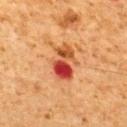Recorded during total-body skin imaging; not selected for excision or biopsy. Cropped from a whole-body photographic skin survey; the tile spans about 15 mm. The lesion is on the back. An algorithmic analysis of the crop reported a lesion area of about 11 mm², an eccentricity of roughly 0.75, and a shape-asymmetry score of about 0.25 (0 = symmetric). And it measured a within-lesion color-variation index near 10/10 and a peripheral color-asymmetry measure near 7.5. A male patient approximately 60 years of age. The tile uses cross-polarized illumination. Longest diameter approximately 4.5 mm.